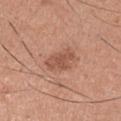lesion_size:
  long_diameter_mm_approx: 4.0
site: right upper arm
automated_metrics:
  area_mm2_approx: 7.0
  eccentricity: 0.8
  shape_asymmetry: 0.2
  cielab_L: 53
  cielab_a: 24
  cielab_b: 30
lighting: white-light
image:
  source: total-body photography crop
  field_of_view_mm: 15
patient:
  sex: male
  age_approx: 35